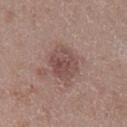This lesion was catalogued during total-body skin photography and was not selected for biopsy. Automated image analysis of the tile measured a border-irregularity rating of about 1.5/10, a color-variation rating of about 4/10, and a peripheral color-asymmetry measure near 1.5. The analysis additionally found an automated nevus-likeness rating near 40 out of 100. Measured at roughly 4 mm in maximum diameter. Imaged with white-light lighting. The patient is a male aged approximately 50. Cropped from a whole-body photographic skin survey; the tile spans about 15 mm. The lesion is located on the left lower leg.